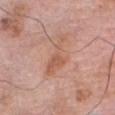{
  "biopsy_status": "not biopsied; imaged during a skin examination",
  "patient": {
    "sex": "male",
    "age_approx": 80
  },
  "site": "head or neck",
  "image": {
    "source": "total-body photography crop",
    "field_of_view_mm": 15
  }
}